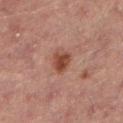Assessment: Part of a total-body skin-imaging series; this lesion was reviewed on a skin check and was not flagged for biopsy. Context: A region of skin cropped from a whole-body photographic capture, roughly 15 mm wide. Located on the left lower leg. Measured at roughly 2.5 mm in maximum diameter. Captured under cross-polarized illumination. The subject is a female aged approximately 70.